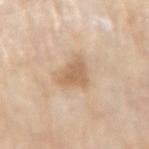Clinical impression: No biopsy was performed on this lesion — it was imaged during a full skin examination and was not determined to be concerning. Acquisition and patient details: A 15 mm crop from a total-body photograph taken for skin-cancer surveillance. An algorithmic analysis of the crop reported border irregularity of about 4.5 on a 0–10 scale, a within-lesion color-variation index near 2/10, and a peripheral color-asymmetry measure near 1. The analysis additionally found a classifier nevus-likeness of about 15/100. Longest diameter approximately 4 mm. The subject is a male in their 80s. Imaged with white-light lighting. The lesion is located on the right upper arm.